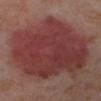{"biopsy_status": "not biopsied; imaged during a skin examination", "site": "left lower leg", "patient": {"sex": "female", "age_approx": 50}, "lighting": "cross-polarized", "image": {"source": "total-body photography crop", "field_of_view_mm": 15}}